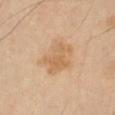A close-up tile cropped from a whole-body skin photograph, about 15 mm across.
The recorded lesion diameter is about 5 mm.
A male patient, aged 63 to 67.
An algorithmic analysis of the crop reported an average lesion color of about L≈64 a*≈18 b*≈36 (CIELAB), about 8 CIELAB-L* units darker than the surrounding skin, and a lesion-to-skin contrast of about 5.5 (normalized; higher = more distinct).
On the right thigh.